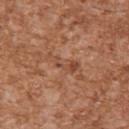{"lesion_size": {"long_diameter_mm_approx": 4.5}, "site": "right upper arm", "image": {"source": "total-body photography crop", "field_of_view_mm": 15}, "lighting": "white-light", "patient": {"sex": "male", "age_approx": 45}}